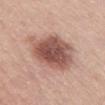Part of a total-body skin-imaging series; this lesion was reviewed on a skin check and was not flagged for biopsy. A male subject in their 70s. A close-up tile cropped from a whole-body skin photograph, about 15 mm across. The lesion is located on the lower back. Automated tile analysis of the lesion measured a lesion area of about 24 mm², an eccentricity of roughly 0.6, and a symmetry-axis asymmetry near 0.2. And it measured a lesion color around L≈53 a*≈22 b*≈25 in CIELAB, a lesion–skin lightness drop of about 15, and a normalized border contrast of about 10. The analysis additionally found a border-irregularity index near 2.5/10 and peripheral color asymmetry of about 1.5. About 7 mm across.